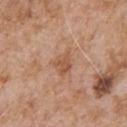biopsy_status: not biopsied; imaged during a skin examination
site: front of the torso
lesion_size:
  long_diameter_mm_approx: 3.5
patient:
  sex: male
  age_approx: 65
lighting: white-light
image:
  source: total-body photography crop
  field_of_view_mm: 15